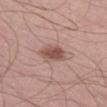<tbp_lesion>
  <biopsy_status>not biopsied; imaged during a skin examination</biopsy_status>
  <patient>
    <sex>male</sex>
    <age_approx>55</age_approx>
  </patient>
  <image>
    <source>total-body photography crop</source>
    <field_of_view_mm>15</field_of_view_mm>
  </image>
  <site>left thigh</site>
</tbp_lesion>